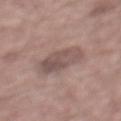Recorded during total-body skin imaging; not selected for excision or biopsy. The lesion's longest dimension is about 5 mm. The lesion is on the mid back. A male subject, in their mid-50s. A roughly 15 mm field-of-view crop from a total-body skin photograph. Captured under white-light illumination.